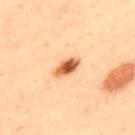Recorded during total-body skin imaging; not selected for excision or biopsy.
An algorithmic analysis of the crop reported about 18 CIELAB-L* units darker than the surrounding skin and a lesion-to-skin contrast of about 11.5 (normalized; higher = more distinct). And it measured an automated nevus-likeness rating near 100 out of 100 and lesion-presence confidence of about 100/100.
The lesion is on the back.
The patient is a male aged around 40.
This is a cross-polarized tile.
A close-up tile cropped from a whole-body skin photograph, about 15 mm across.
The recorded lesion diameter is about 3.5 mm.